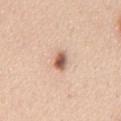Captured during whole-body skin photography for melanoma surveillance; the lesion was not biopsied. The lesion is located on the chest. A 15 mm crop from a total-body photograph taken for skin-cancer surveillance. A male patient, about 45 years old. The recorded lesion diameter is about 3 mm. Imaged with white-light lighting. The lesion-visualizer software estimated an area of roughly 4 mm². It also reported a mean CIELAB color near L≈60 a*≈21 b*≈29, roughly 17 lightness units darker than nearby skin, and a normalized lesion–skin contrast near 10. The analysis additionally found a border-irregularity rating of about 2.5/10 and radial color variation of about 1.5. It also reported an automated nevus-likeness rating near 100 out of 100 and lesion-presence confidence of about 100/100.